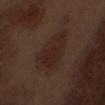Impression: Imaged during a routine full-body skin examination; the lesion was not biopsied and no histopathology is available.